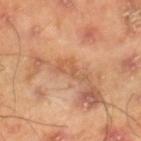Impression: Part of a total-body skin-imaging series; this lesion was reviewed on a skin check and was not flagged for biopsy.